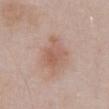biopsy_status: not biopsied; imaged during a skin examination
lesion_size:
  long_diameter_mm_approx: 4.0
automated_metrics:
  area_mm2_approx: 9.0
  eccentricity: 0.6
  shape_asymmetry: 0.35
  border_irregularity_0_10: 4.0
  color_variation_0_10: 3.0
  peripheral_color_asymmetry: 1.0
  lesion_detection_confidence_0_100: 100
site: abdomen
lighting: white-light
patient:
  sex: male
  age_approx: 55
image:
  source: total-body photography crop
  field_of_view_mm: 15